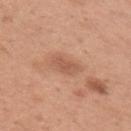Q: Was a biopsy performed?
A: total-body-photography surveillance lesion; no biopsy
Q: How large is the lesion?
A: ≈3.5 mm
Q: Where on the body is the lesion?
A: the arm
Q: Who is the patient?
A: female, approximately 30 years of age
Q: How was this image acquired?
A: ~15 mm tile from a whole-body skin photo
Q: How was the tile lit?
A: white-light illumination
Q: What did automated image analysis measure?
A: a border-irregularity rating of about 3/10, internal color variation of about 2 on a 0–10 scale, and radial color variation of about 0.5; an automated nevus-likeness rating near 0 out of 100